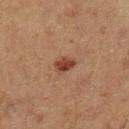Assessment:
The lesion was photographed on a routine skin check and not biopsied; there is no pathology result.
Image and clinical context:
Automated tile analysis of the lesion measured a footprint of about 4 mm², a shape eccentricity near 0.65, and two-axis asymmetry of about 0.3. And it measured a mean CIELAB color near L≈34 a*≈19 b*≈25, roughly 10 lightness units darker than nearby skin, and a lesion-to-skin contrast of about 9 (normalized; higher = more distinct). The software also gave internal color variation of about 2.5 on a 0–10 scale and peripheral color asymmetry of about 1. And it measured a lesion-detection confidence of about 100/100. The tile uses cross-polarized illumination. A roughly 15 mm field-of-view crop from a total-body skin photograph. The lesion is on the left lower leg. A female subject approximately 50 years of age.